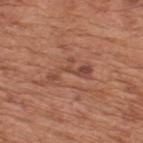| field | value |
|---|---|
| notes | no biopsy performed (imaged during a skin exam) |
| illumination | white-light illumination |
| patient | male, aged 63–67 |
| imaging modality | ~15 mm tile from a whole-body skin photo |
| body site | the upper back |
| lesion diameter | about 5 mm |